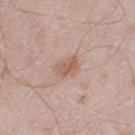biopsy status = catalogued during a skin exam; not biopsied
image source = total-body-photography crop, ~15 mm field of view
diameter = ~2.5 mm (longest diameter)
subject = male, aged around 60
automated metrics = a lesion area of about 4.5 mm², an eccentricity of roughly 0.7, and a symmetry-axis asymmetry near 0.25; a classifier nevus-likeness of about 65/100 and lesion-presence confidence of about 100/100
location = the right thigh